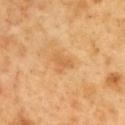workup: no biopsy performed (imaged during a skin exam)
anatomic site: the mid back
lesion diameter: ≈2.5 mm
automated metrics: a lesion area of about 3 mm², a shape eccentricity near 0.8, and a symmetry-axis asymmetry near 0.6; border irregularity of about 5.5 on a 0–10 scale; a nevus-likeness score of about 0/100 and a lesion-detection confidence of about 100/100
tile lighting: cross-polarized
imaging modality: ~15 mm tile from a whole-body skin photo
subject: male, aged approximately 50